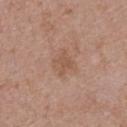Q: What is the imaging modality?
A: ~15 mm crop, total-body skin-cancer survey
Q: What are the patient's age and sex?
A: female, approximately 30 years of age
Q: What is the anatomic site?
A: the upper back
Q: Illumination type?
A: white-light